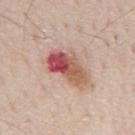The lesion was photographed on a routine skin check and not biopsied; there is no pathology result. A male subject aged 78 to 82. The tile uses white-light illumination. Measured at roughly 5.5 mm in maximum diameter. From the mid back. A lesion tile, about 15 mm wide, cut from a 3D total-body photograph. The lesion-visualizer software estimated an eccentricity of roughly 0.85 and a symmetry-axis asymmetry near 0.25. It also reported a lesion color around L≈55 a*≈26 b*≈27 in CIELAB, roughly 15 lightness units darker than nearby skin, and a normalized border contrast of about 10. And it measured internal color variation of about 10 on a 0–10 scale and peripheral color asymmetry of about 7. The software also gave a detector confidence of about 100 out of 100 that the crop contains a lesion.